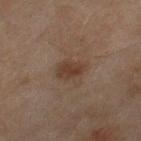Notes:
- workup — imaged on a skin check; not biopsied
- lesion diameter — about 3 mm
- automated metrics — an outline eccentricity of about 0.75 (0 = round, 1 = elongated) and a shape-asymmetry score of about 0.2 (0 = symmetric); a lesion-detection confidence of about 100/100
- patient — female, aged around 60
- tile lighting — cross-polarized illumination
- body site — the right thigh
- acquisition — ~15 mm crop, total-body skin-cancer survey